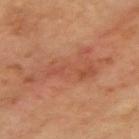Clinical impression: This lesion was catalogued during total-body skin photography and was not selected for biopsy. Acquisition and patient details: About 9.5 mm across. From the left arm. Imaged with cross-polarized lighting. Automated tile analysis of the lesion measured radial color variation of about 1. The software also gave a nevus-likeness score of about 0/100 and lesion-presence confidence of about 100/100. A 15 mm close-up tile from a total-body photography series done for melanoma screening. A male patient, about 65 years old.